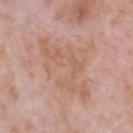The lesion was photographed on a routine skin check and not biopsied; there is no pathology result. From the head or neck. A male subject aged 68–72. The lesion-visualizer software estimated an outline eccentricity of about 0.65 (0 = round, 1 = elongated). The software also gave a lesion color around L≈61 a*≈20 b*≈29 in CIELAB, roughly 6 lightness units darker than nearby skin, and a normalized border contrast of about 5.5. And it measured a border-irregularity index near 6/10 and a within-lesion color-variation index near 4/10. The software also gave a classifier nevus-likeness of about 0/100 and a detector confidence of about 95 out of 100 that the crop contains a lesion. A 15 mm close-up extracted from a 3D total-body photography capture. The tile uses white-light illumination.